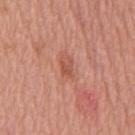{"biopsy_status": "not biopsied; imaged during a skin examination", "patient": {"sex": "male", "age_approx": 65}, "site": "mid back", "image": {"source": "total-body photography crop", "field_of_view_mm": 15}, "lighting": "white-light", "lesion_size": {"long_diameter_mm_approx": 2.5}}